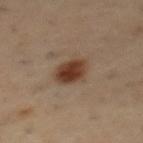{"patient": {"sex": "male", "age_approx": 55}, "image": {"source": "total-body photography crop", "field_of_view_mm": 15}, "lesion_size": {"long_diameter_mm_approx": 4.0}, "site": "mid back", "lighting": "cross-polarized"}